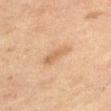body site = the right thigh; illumination = cross-polarized; subject = female, about 60 years old; acquisition = 15 mm crop, total-body photography; lesion diameter = ~3.5 mm (longest diameter).